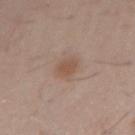  biopsy_status: not biopsied; imaged during a skin examination
  patient:
    sex: male
    age_approx: 30
  automated_metrics:
    cielab_L: 52
    cielab_a: 18
    cielab_b: 27
    vs_skin_darker_L: 8.0
    vs_skin_contrast_norm: 6.5
    border_irregularity_0_10: 2.0
    nevus_likeness_0_100: 85
  image:
    source: total-body photography crop
    field_of_view_mm: 15
  site: right upper arm
  lighting: white-light